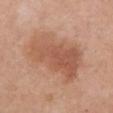The lesion was photographed on a routine skin check and not biopsied; there is no pathology result. The lesion-visualizer software estimated a nevus-likeness score of about 20/100. A female subject in their mid- to late 50s. A 15 mm close-up tile from a total-body photography series done for melanoma screening. From the chest. The tile uses white-light illumination.